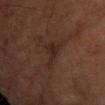Clinical impression: The lesion was photographed on a routine skin check and not biopsied; there is no pathology result. Clinical summary: The lesion is located on the left forearm. A male subject, aged around 60. This image is a 15 mm lesion crop taken from a total-body photograph. The tile uses cross-polarized illumination.